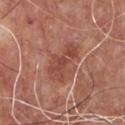biopsy status: no biopsy performed (imaged during a skin exam); automated lesion analysis: a footprint of about 9.5 mm² and an outline eccentricity of about 0.9 (0 = round, 1 = elongated); image: 15 mm crop, total-body photography; location: the chest; diameter: ≈5 mm; tile lighting: white-light illumination; subject: male, aged around 65.